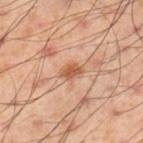• workup · no biopsy performed (imaged during a skin exam)
• diameter · ~2.5 mm (longest diameter)
• image-analysis metrics · an average lesion color of about L≈58 a*≈24 b*≈36 (CIELAB), a lesion–skin lightness drop of about 11, and a lesion-to-skin contrast of about 7.5 (normalized; higher = more distinct); a border-irregularity index near 2/10 and a peripheral color-asymmetry measure near 1
• patient · male, aged around 55
• acquisition · total-body-photography crop, ~15 mm field of view
• lighting · cross-polarized
• body site · the right lower leg